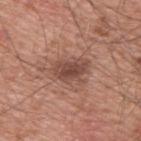Background: The lesion-visualizer software estimated an eccentricity of roughly 0.75 and two-axis asymmetry of about 0.3. The software also gave a nevus-likeness score of about 45/100 and a lesion-detection confidence of about 100/100. Cropped from a total-body skin-imaging series; the visible field is about 15 mm. Imaged with white-light lighting. From the upper back. The subject is a male in their mid-50s.